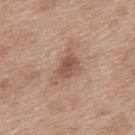Impression:
Captured during whole-body skin photography for melanoma surveillance; the lesion was not biopsied.
Context:
Automated image analysis of the tile measured a mean CIELAB color near L≈54 a*≈19 b*≈29, about 10 CIELAB-L* units darker than the surrounding skin, and a normalized lesion–skin contrast near 7. It also reported a border-irregularity rating of about 5.5/10, internal color variation of about 3.5 on a 0–10 scale, and peripheral color asymmetry of about 1. The software also gave a classifier nevus-likeness of about 10/100. The lesion is on the upper back. A region of skin cropped from a whole-body photographic capture, roughly 15 mm wide. This is a white-light tile. The subject is a female aged around 40. Approximately 4.5 mm at its widest.